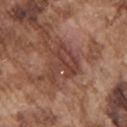notes: catalogued during a skin exam; not biopsied
patient: male, aged approximately 75
site: the chest
tile lighting: white-light
automated metrics: a shape eccentricity near 0.6 and a shape-asymmetry score of about 0.6 (0 = symmetric); a border-irregularity rating of about 8/10, internal color variation of about 3.5 on a 0–10 scale, and radial color variation of about 1
image source: 15 mm crop, total-body photography